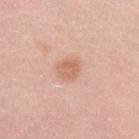The lesion was tiled from a total-body skin photograph and was not biopsied.
Imaged with white-light lighting.
A 15 mm crop from a total-body photograph taken for skin-cancer surveillance.
Longest diameter approximately 2.5 mm.
The subject is a male roughly 30 years of age.